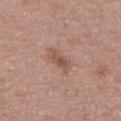The lesion was tiled from a total-body skin photograph and was not biopsied.
The lesion is located on the chest.
Captured under white-light illumination.
Cropped from a total-body skin-imaging series; the visible field is about 15 mm.
Approximately 3.5 mm at its widest.
A female patient, about 50 years old.